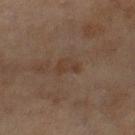The lesion was tiled from a total-body skin photograph and was not biopsied. A roughly 15 mm field-of-view crop from a total-body skin photograph. Located on the right thigh. Imaged with cross-polarized lighting. Approximately 3 mm at its widest. The lesion-visualizer software estimated a mean CIELAB color near L≈34 a*≈14 b*≈24, a lesion–skin lightness drop of about 5, and a normalized border contrast of about 5. It also reported a border-irregularity rating of about 4/10 and internal color variation of about 2.5 on a 0–10 scale. A female subject aged 58 to 62.